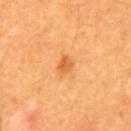biopsy_status: not biopsied; imaged during a skin examination
lighting: cross-polarized
patient:
  sex: male
  age_approx: 60
lesion_size:
  long_diameter_mm_approx: 2.0
image:
  source: total-body photography crop
  field_of_view_mm: 15
site: mid back